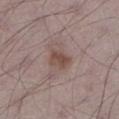The lesion was tiled from a total-body skin photograph and was not biopsied. The tile uses white-light illumination. The subject is a male aged approximately 70. On the left lower leg. A 15 mm close-up extracted from a 3D total-body photography capture. Automated tile analysis of the lesion measured a lesion area of about 5.5 mm², an outline eccentricity of about 0.6 (0 = round, 1 = elongated), and a shape-asymmetry score of about 0.2 (0 = symmetric). The analysis additionally found a lesion color around L≈48 a*≈17 b*≈22 in CIELAB and a normalized lesion–skin contrast near 7.5. It also reported a border-irregularity index near 2/10 and a within-lesion color-variation index near 2.5/10. It also reported an automated nevus-likeness rating near 60 out of 100 and a lesion-detection confidence of about 100/100.